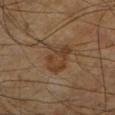Impression:
No biopsy was performed on this lesion — it was imaged during a full skin examination and was not determined to be concerning.
Context:
Measured at roughly 4.5 mm in maximum diameter. The subject is a male aged 63–67. From the left lower leg. Captured under cross-polarized illumination. A 15 mm close-up extracted from a 3D total-body photography capture.